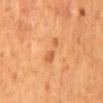Clinical impression: Imaged during a routine full-body skin examination; the lesion was not biopsied and no histopathology is available. Clinical summary: The lesion is located on the mid back. Cropped from a total-body skin-imaging series; the visible field is about 15 mm. A male subject in their mid- to late 50s. Approximately 4 mm at its widest.